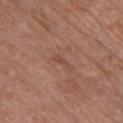Captured during whole-body skin photography for melanoma surveillance; the lesion was not biopsied.
The patient is a female in their mid- to late 70s.
Measured at roughly 2.5 mm in maximum diameter.
A region of skin cropped from a whole-body photographic capture, roughly 15 mm wide.
Automated tile analysis of the lesion measured a footprint of about 2 mm², a shape eccentricity near 0.95, and two-axis asymmetry of about 0.4. The analysis additionally found an average lesion color of about L≈47 a*≈21 b*≈28 (CIELAB) and a normalized lesion–skin contrast near 5.
On the chest.